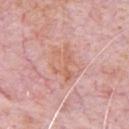notes=catalogued during a skin exam; not biopsied
patient=male, aged 78–82
body site=the chest
image=~15 mm crop, total-body skin-cancer survey